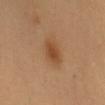This lesion was catalogued during total-body skin photography and was not selected for biopsy. Approximately 4 mm at its widest. A close-up tile cropped from a whole-body skin photograph, about 15 mm across. The lesion is on the mid back. A female patient, about 60 years old. Automated tile analysis of the lesion measured a footprint of about 7 mm², a shape eccentricity near 0.85, and a shape-asymmetry score of about 0.2 (0 = symmetric). And it measured a lesion color around L≈49 a*≈21 b*≈36 in CIELAB, a lesion–skin lightness drop of about 9, and a lesion-to-skin contrast of about 7 (normalized; higher = more distinct). It also reported a classifier nevus-likeness of about 100/100 and a detector confidence of about 100 out of 100 that the crop contains a lesion.